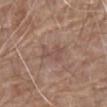Imaged during a routine full-body skin examination; the lesion was not biopsied and no histopathology is available. The patient is a male in their 80s. About 3 mm across. Located on the right upper arm. A roughly 15 mm field-of-view crop from a total-body skin photograph.